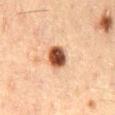{
  "patient": {
    "sex": "male",
    "age_approx": 50
  },
  "image": {
    "source": "total-body photography crop",
    "field_of_view_mm": 15
  },
  "site": "lower back",
  "lesion_size": {
    "long_diameter_mm_approx": 3.0
  }
}